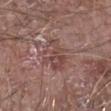Located on the arm.
A male subject, approximately 80 years of age.
A region of skin cropped from a whole-body photographic capture, roughly 15 mm wide.